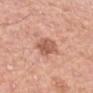notes — no biopsy performed (imaged during a skin exam)
TBP lesion metrics — a mean CIELAB color near L≈58 a*≈25 b*≈30 and a lesion–skin lightness drop of about 11; a border-irregularity rating of about 2.5/10, a color-variation rating of about 3/10, and peripheral color asymmetry of about 1
imaging modality — ~15 mm crop, total-body skin-cancer survey
lighting — white-light
body site — the left upper arm
subject — female, aged around 50
lesion diameter — about 4 mm